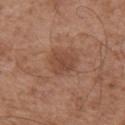On the left upper arm.
The subject is a male aged around 50.
Measured at roughly 3 mm in maximum diameter.
A 15 mm close-up tile from a total-body photography series done for melanoma screening.
Automated tile analysis of the lesion measured a classifier nevus-likeness of about 25/100 and a lesion-detection confidence of about 100/100.
Imaged with white-light lighting.